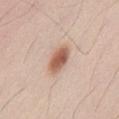<record>
<biopsy_status>not biopsied; imaged during a skin examination</biopsy_status>
<patient>
  <sex>male</sex>
  <age_approx>35</age_approx>
</patient>
<lesion_size>
  <long_diameter_mm_approx>4.0</long_diameter_mm_approx>
</lesion_size>
<site>front of the torso</site>
<image>
  <source>total-body photography crop</source>
  <field_of_view_mm>15</field_of_view_mm>
</image>
<lighting>white-light</lighting>
</record>